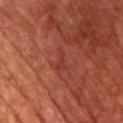Clinical impression:
Part of a total-body skin-imaging series; this lesion was reviewed on a skin check and was not flagged for biopsy.
Clinical summary:
A 15 mm close-up extracted from a 3D total-body photography capture. Approximately 2.5 mm at its widest. A male subject aged 68–72. On the chest. The tile uses cross-polarized illumination.